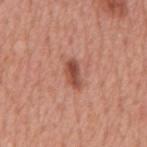image — 15 mm crop, total-body photography; lighting — white-light; anatomic site — the mid back; diameter — ~3.5 mm (longest diameter); subject — male, in their mid- to late 60s.